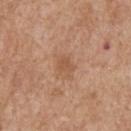Imaged during a routine full-body skin examination; the lesion was not biopsied and no histopathology is available.
Captured under white-light illumination.
About 2.5 mm across.
The lesion-visualizer software estimated a border-irregularity index near 3.5/10, a within-lesion color-variation index near 1/10, and radial color variation of about 0.5.
A male subject, aged 58–62.
The lesion is located on the back.
A region of skin cropped from a whole-body photographic capture, roughly 15 mm wide.